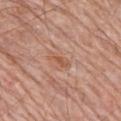| feature | finding |
|---|---|
| notes | total-body-photography surveillance lesion; no biopsy |
| TBP lesion metrics | a lesion–skin lightness drop of about 7 |
| size | ≈2.5 mm |
| patient | male, aged approximately 80 |
| tile lighting | white-light illumination |
| location | the back |
| imaging modality | total-body-photography crop, ~15 mm field of view |